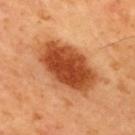Assessment:
Recorded during total-body skin imaging; not selected for excision or biopsy.
Context:
Located on the upper back. Imaged with cross-polarized lighting. The lesion's longest dimension is about 7.5 mm. Cropped from a total-body skin-imaging series; the visible field is about 15 mm. A male patient, aged 63–67. The total-body-photography lesion software estimated an eccentricity of roughly 0.8 and a symmetry-axis asymmetry near 0.15. The software also gave a mean CIELAB color near L≈49 a*≈31 b*≈41 and a normalized border contrast of about 11. It also reported a border-irregularity rating of about 2/10, a within-lesion color-variation index near 5/10, and peripheral color asymmetry of about 1.5.